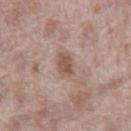Q: Is there a histopathology result?
A: no biopsy performed (imaged during a skin exam)
Q: Who is the patient?
A: male, aged around 70
Q: What is the anatomic site?
A: the front of the torso
Q: Illumination type?
A: white-light illumination
Q: Lesion size?
A: ≈2.5 mm
Q: What is the imaging modality?
A: total-body-photography crop, ~15 mm field of view